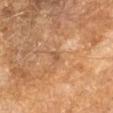Captured during whole-body skin photography for melanoma surveillance; the lesion was not biopsied.
The patient is a female aged around 65.
Cropped from a whole-body photographic skin survey; the tile spans about 15 mm.
On the left forearm.